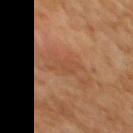Case summary:
• image-analysis metrics — a footprint of about 4.5 mm², an eccentricity of roughly 0.95, and two-axis asymmetry of about 0.45; a mean CIELAB color near L≈47 a*≈22 b*≈34, roughly 6 lightness units darker than nearby skin, and a normalized border contrast of about 4.5; a border-irregularity rating of about 6/10, internal color variation of about 1 on a 0–10 scale, and radial color variation of about 0.5
• image — total-body-photography crop, ~15 mm field of view
• patient — female, aged around 50
• location — the upper back
• lighting — cross-polarized
• lesion size — ≈4.5 mm